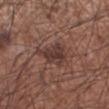Q: Was a biopsy performed?
A: total-body-photography surveillance lesion; no biopsy
Q: Lesion size?
A: ≈5 mm
Q: What lighting was used for the tile?
A: white-light illumination
Q: What is the imaging modality?
A: ~15 mm tile from a whole-body skin photo
Q: Where on the body is the lesion?
A: the arm
Q: Patient demographics?
A: male, in their mid-50s